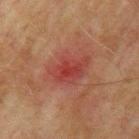Clinical impression:
The lesion was tiled from a total-body skin photograph and was not biopsied.
Background:
About 5 mm across. Cropped from a total-body skin-imaging series; the visible field is about 15 mm. On the left upper arm. A male patient in their mid- to late 70s.